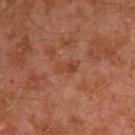Impression:
Part of a total-body skin-imaging series; this lesion was reviewed on a skin check and was not flagged for biopsy.
Context:
The total-body-photography lesion software estimated a border-irregularity rating of about 3.5/10. A 15 mm crop from a total-body photograph taken for skin-cancer surveillance. Approximately 3 mm at its widest. A male patient, roughly 30 years of age. On the left arm.